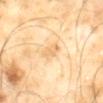This lesion was catalogued during total-body skin photography and was not selected for biopsy.
Automated image analysis of the tile measured a mean CIELAB color near L≈71 a*≈16 b*≈41, a lesion–skin lightness drop of about 8, and a normalized lesion–skin contrast near 5.5. And it measured a border-irregularity index near 3/10, a within-lesion color-variation index near 1.5/10, and a peripheral color-asymmetry measure near 0.5.
Imaged with cross-polarized lighting.
The lesion is located on the mid back.
A 15 mm close-up extracted from a 3D total-body photography capture.
The subject is a male about 65 years old.